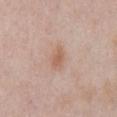follow-up = catalogued during a skin exam; not biopsied
body site = the abdomen
lesion diameter = about 2.5 mm
subject = male, approximately 55 years of age
illumination = white-light
image = total-body-photography crop, ~15 mm field of view